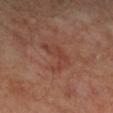biopsy status: imaged on a skin check; not biopsied | imaging modality: ~15 mm tile from a whole-body skin photo | subject: male, approximately 50 years of age | site: the left leg | lesion diameter: about 4 mm.